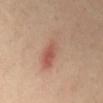{"biopsy_status": "not biopsied; imaged during a skin examination", "image": {"source": "total-body photography crop", "field_of_view_mm": 15}, "patient": {"sex": "female", "age_approx": 40}, "site": "mid back", "automated_metrics": {"eccentricity": 0.95, "shape_asymmetry": 0.25, "vs_skin_darker_L": 9.0, "vs_skin_contrast_norm": 6.0, "nevus_likeness_0_100": 45, "lesion_detection_confidence_0_100": 100}, "lesion_size": {"long_diameter_mm_approx": 6.0}, "lighting": "cross-polarized"}